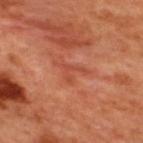follow-up — catalogued during a skin exam; not biopsied | body site — the upper back | image source — ~15 mm tile from a whole-body skin photo | subject — male, aged 48 to 52 | lesion diameter — about 4 mm | illumination — cross-polarized.